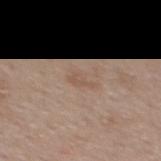workup = catalogued during a skin exam; not biopsied | subject = female, about 40 years old | automated metrics = border irregularity of about 5.5 on a 0–10 scale, a within-lesion color-variation index near 0/10, and radial color variation of about 0; a nevus-likeness score of about 0/100 | body site = the upper back | lesion diameter = ~3 mm (longest diameter) | tile lighting = white-light illumination | image = ~15 mm tile from a whole-body skin photo.